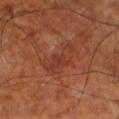From the leg.
The tile uses cross-polarized illumination.
A close-up tile cropped from a whole-body skin photograph, about 15 mm across.
The lesion's longest dimension is about 5.5 mm.
The subject is a male in their 70s.